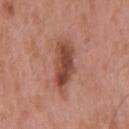<case>
<biopsy_status>not biopsied; imaged during a skin examination</biopsy_status>
<image>
  <source>total-body photography crop</source>
  <field_of_view_mm>15</field_of_view_mm>
</image>
<patient>
  <sex>male</sex>
  <age_approx>55</age_approx>
</patient>
<lesion_size>
  <long_diameter_mm_approx>6.0</long_diameter_mm_approx>
</lesion_size>
<automated_metrics>
  <border_irregularity_0_10>3.0</border_irregularity_0_10>
  <color_variation_0_10>5.0</color_variation_0_10>
  <lesion_detection_confidence_0_100>100</lesion_detection_confidence_0_100>
</automated_metrics>
<lighting>white-light</lighting>
<site>mid back</site>
</case>